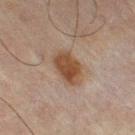follow-up: no biopsy performed (imaged during a skin exam) | imaging modality: ~15 mm tile from a whole-body skin photo | site: the left thigh | subject: male, about 65 years old | tile lighting: cross-polarized | TBP lesion metrics: border irregularity of about 2 on a 0–10 scale, internal color variation of about 3.5 on a 0–10 scale, and a peripheral color-asymmetry measure near 1; a classifier nevus-likeness of about 90/100 and lesion-presence confidence of about 100/100.